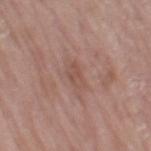| feature | finding |
|---|---|
| notes | total-body-photography surveillance lesion; no biopsy |
| image source | total-body-photography crop, ~15 mm field of view |
| subject | female, aged 68–72 |
| lesion size | ~3 mm (longest diameter) |
| tile lighting | white-light illumination |
| anatomic site | the right thigh |